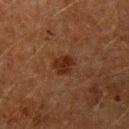| feature | finding |
|---|---|
| subject | male, aged around 60 |
| lesion diameter | ~2.5 mm (longest diameter) |
| imaging modality | ~15 mm crop, total-body skin-cancer survey |
| body site | the left upper arm |
| automated lesion analysis | lesion-presence confidence of about 100/100 |